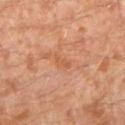Impression:
Captured during whole-body skin photography for melanoma surveillance; the lesion was not biopsied.
Background:
The tile uses cross-polarized illumination. This image is a 15 mm lesion crop taken from a total-body photograph. Automated tile analysis of the lesion measured a footprint of about 2.5 mm², an outline eccentricity of about 0.9 (0 = round, 1 = elongated), and a symmetry-axis asymmetry near 0.4. The analysis additionally found border irregularity of about 4 on a 0–10 scale and a color-variation rating of about 0/10. It also reported an automated nevus-likeness rating near 0 out of 100. A male patient, aged approximately 30. Approximately 2.5 mm at its widest. On the left lower leg.